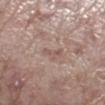Clinical impression: The lesion was tiled from a total-body skin photograph and was not biopsied. Context: A male patient aged around 70. From the right lower leg. This image is a 15 mm lesion crop taken from a total-body photograph. Approximately 2.5 mm at its widest.